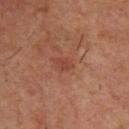Q: Was this lesion biopsied?
A: no biopsy performed (imaged during a skin exam)
Q: What lighting was used for the tile?
A: cross-polarized
Q: Lesion location?
A: the upper back
Q: What are the patient's age and sex?
A: male, approximately 55 years of age
Q: What did automated image analysis measure?
A: a footprint of about 3 mm²; a nevus-likeness score of about 5/100
Q: How was this image acquired?
A: ~15 mm crop, total-body skin-cancer survey